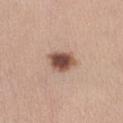Q: Was a biopsy performed?
A: imaged on a skin check; not biopsied
Q: Lesion size?
A: ≈3.5 mm
Q: Patient demographics?
A: female, approximately 40 years of age
Q: Automated lesion metrics?
A: a mean CIELAB color near L≈51 a*≈20 b*≈27 and a lesion-to-skin contrast of about 11.5 (normalized; higher = more distinct); a border-irregularity index near 2.5/10, internal color variation of about 4.5 on a 0–10 scale, and a peripheral color-asymmetry measure near 1; lesion-presence confidence of about 100/100
Q: What kind of image is this?
A: ~15 mm crop, total-body skin-cancer survey
Q: What is the anatomic site?
A: the abdomen